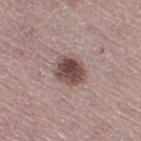Recorded during total-body skin imaging; not selected for excision or biopsy. Cropped from a total-body skin-imaging series; the visible field is about 15 mm. The lesion is on the right thigh. Automated tile analysis of the lesion measured a mean CIELAB color near L≈47 a*≈18 b*≈20, about 14 CIELAB-L* units darker than the surrounding skin, and a lesion-to-skin contrast of about 10.5 (normalized; higher = more distinct). The analysis additionally found a color-variation rating of about 5.5/10 and radial color variation of about 1.5. Approximately 4 mm at its widest. A female subject, aged around 50.